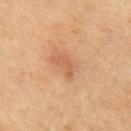Assessment: Imaged during a routine full-body skin examination; the lesion was not biopsied and no histopathology is available. Clinical summary: The subject is a male aged 73 to 77. The tile uses cross-polarized illumination. Approximately 3 mm at its widest. A 15 mm crop from a total-body photograph taken for skin-cancer surveillance. An algorithmic analysis of the crop reported a border-irregularity index near 5/10, a within-lesion color-variation index near 0/10, and radial color variation of about 0. From the chest.